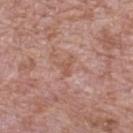Imaged during a routine full-body skin examination; the lesion was not biopsied and no histopathology is available.
The lesion's longest dimension is about 3 mm.
On the mid back.
Cropped from a total-body skin-imaging series; the visible field is about 15 mm.
A male patient aged around 70.